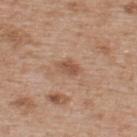{"biopsy_status": "not biopsied; imaged during a skin examination", "patient": {"sex": "male", "age_approx": 65}, "automated_metrics": {"cielab_L": 52, "cielab_a": 21, "cielab_b": 31, "vs_skin_darker_L": 9.0, "vs_skin_contrast_norm": 7.0}, "site": "upper back", "image": {"source": "total-body photography crop", "field_of_view_mm": 15}, "lighting": "white-light"}